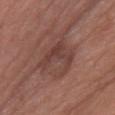notes: catalogued during a skin exam; not biopsied
lesion size: ~6 mm (longest diameter)
image source: ~15 mm tile from a whole-body skin photo
subject: female, aged approximately 70
illumination: white-light illumination
anatomic site: the chest
TBP lesion metrics: an area of roughly 19 mm² and a shape eccentricity near 0.7; a mean CIELAB color near L≈42 a*≈20 b*≈23 and a normalized border contrast of about 6; a border-irregularity index near 3/10 and internal color variation of about 5.5 on a 0–10 scale; a classifier nevus-likeness of about 15/100